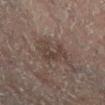{
  "biopsy_status": "not biopsied; imaged during a skin examination",
  "patient": {
    "sex": "female",
    "age_approx": 80
  },
  "image": {
    "source": "total-body photography crop",
    "field_of_view_mm": 15
  },
  "site": "leg",
  "lesion_size": {
    "long_diameter_mm_approx": 4.0
  }
}